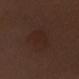Imaged during a routine full-body skin examination; the lesion was not biopsied and no histopathology is available. A close-up tile cropped from a whole-body skin photograph, about 15 mm across. From the arm. Imaged with white-light lighting. Approximately 5 mm at its widest. The subject is a female aged around 30.